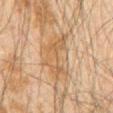Impression:
The lesion was tiled from a total-body skin photograph and was not biopsied.
Acquisition and patient details:
A male subject, aged approximately 60. A close-up tile cropped from a whole-body skin photograph, about 15 mm across. About 6.5 mm across. Imaged with cross-polarized lighting. The lesion is located on the abdomen. Automated tile analysis of the lesion measured an outline eccentricity of about 0.75 (0 = round, 1 = elongated). And it measured border irregularity of about 5 on a 0–10 scale. It also reported a nevus-likeness score of about 0/100 and lesion-presence confidence of about 80/100.